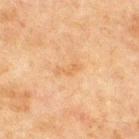This lesion was catalogued during total-body skin photography and was not selected for biopsy.
Automated image analysis of the tile measured a shape eccentricity near 0.9 and a symmetry-axis asymmetry near 0.4. And it measured a classifier nevus-likeness of about 0/100 and a lesion-detection confidence of about 100/100.
The lesion is located on the chest.
A lesion tile, about 15 mm wide, cut from a 3D total-body photograph.
The subject is a male aged 63 to 67.
The lesion's longest dimension is about 3 mm.